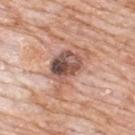follow-up = imaged on a skin check; not biopsied
patient = male, roughly 80 years of age
anatomic site = the upper back
image source = ~15 mm tile from a whole-body skin photo
lesion diameter = ≈6.5 mm